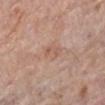Q: Is there a histopathology result?
A: imaged on a skin check; not biopsied
Q: Who is the patient?
A: female, aged 68–72
Q: Lesion size?
A: ~2.5 mm (longest diameter)
Q: Illumination type?
A: white-light
Q: Where on the body is the lesion?
A: the left lower leg
Q: What kind of image is this?
A: total-body-photography crop, ~15 mm field of view